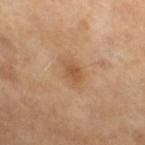workup: catalogued during a skin exam; not biopsied | image source: total-body-photography crop, ~15 mm field of view | lighting: cross-polarized | size: ~3 mm (longest diameter) | TBP lesion metrics: a lesion color around L≈51 a*≈20 b*≈35 in CIELAB, about 8 CIELAB-L* units darker than the surrounding skin, and a normalized lesion–skin contrast near 6.5 | patient: male, aged around 65.